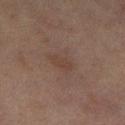diameter = about 3 mm | patient = female, aged 58–62 | site = the leg | lighting = cross-polarized | imaging modality = ~15 mm crop, total-body skin-cancer survey | automated metrics = an automated nevus-likeness rating near 5 out of 100 and a lesion-detection confidence of about 100/100.